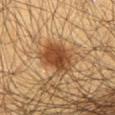Assessment: The lesion was photographed on a routine skin check and not biopsied; there is no pathology result. Clinical summary: A 15 mm close-up tile from a total-body photography series done for melanoma screening. Automated tile analysis of the lesion measured an area of roughly 11 mm², an outline eccentricity of about 0.75 (0 = round, 1 = elongated), and a symmetry-axis asymmetry near 0.25. The analysis additionally found a mean CIELAB color near L≈42 a*≈21 b*≈34, a lesion–skin lightness drop of about 14, and a normalized border contrast of about 10.5. It also reported a nevus-likeness score of about 100/100 and a detector confidence of about 100 out of 100 that the crop contains a lesion. Captured under cross-polarized illumination. The recorded lesion diameter is about 4.5 mm. On the abdomen. A male patient aged around 60.